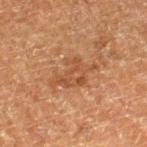Assessment:
The lesion was photographed on a routine skin check and not biopsied; there is no pathology result.
Context:
A roughly 15 mm field-of-view crop from a total-body skin photograph. The lesion is located on the right lower leg. Approximately 5.5 mm at its widest. A male patient about 75 years old. Imaged with cross-polarized lighting.